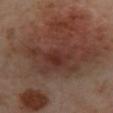Impression: The lesion was photographed on a routine skin check and not biopsied; there is no pathology result. Context: A female subject aged 53 to 57. A lesion tile, about 15 mm wide, cut from a 3D total-body photograph. Imaged with cross-polarized lighting. Located on the left lower leg. Automated tile analysis of the lesion measured an area of roughly 115 mm², an outline eccentricity of about 0.85 (0 = round, 1 = elongated), and a symmetry-axis asymmetry near 0.45. The software also gave a border-irregularity rating of about 8/10, a color-variation rating of about 7.5/10, and peripheral color asymmetry of about 2. The analysis additionally found a classifier nevus-likeness of about 5/100 and a detector confidence of about 100 out of 100 that the crop contains a lesion. Approximately 18.5 mm at its widest.